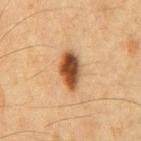The lesion was photographed on a routine skin check and not biopsied; there is no pathology result.
A male patient, in their mid- to late 60s.
Cropped from a total-body skin-imaging series; the visible field is about 15 mm.
Automated image analysis of the tile measured a lesion area of about 9.5 mm², an outline eccentricity of about 0.85 (0 = round, 1 = elongated), and two-axis asymmetry of about 0.3. The software also gave an average lesion color of about L≈42 a*≈19 b*≈32 (CIELAB) and a lesion–skin lightness drop of about 16. It also reported a nevus-likeness score of about 100/100.
From the chest.